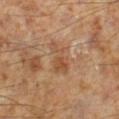Assessment:
Captured during whole-body skin photography for melanoma surveillance; the lesion was not biopsied.
Image and clinical context:
The tile uses cross-polarized illumination. A 15 mm close-up tile from a total-body photography series done for melanoma screening. The lesion-visualizer software estimated border irregularity of about 6 on a 0–10 scale, internal color variation of about 3 on a 0–10 scale, and radial color variation of about 1. It also reported a classifier nevus-likeness of about 0/100 and lesion-presence confidence of about 100/100. Approximately 4 mm at its widest. From the left lower leg. A male patient in their 60s.